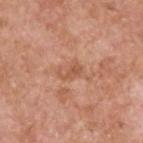The lesion was photographed on a routine skin check and not biopsied; there is no pathology result. The lesion is located on the back. The subject is a male aged 58 to 62. A lesion tile, about 15 mm wide, cut from a 3D total-body photograph.